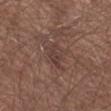<lesion>
<biopsy_status>not biopsied; imaged during a skin examination</biopsy_status>
<site>left forearm</site>
<image>
  <source>total-body photography crop</source>
  <field_of_view_mm>15</field_of_view_mm>
</image>
<patient>
  <sex>male</sex>
  <age_approx>55</age_approx>
</patient>
</lesion>